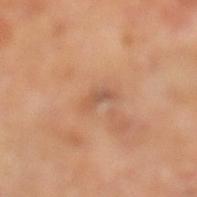automated metrics: a lesion area of about 3 mm², a shape eccentricity near 0.9, and two-axis asymmetry of about 0.5; a mean CIELAB color near L≈56 a*≈22 b*≈33 and a lesion–skin lightness drop of about 8; a nevus-likeness score of about 0/100 and lesion-presence confidence of about 100/100 | location: the left lower leg | acquisition: total-body-photography crop, ~15 mm field of view | size: ~3 mm (longest diameter) | patient: male, roughly 65 years of age.